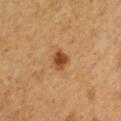workup: imaged on a skin check; not biopsied
subject: male, aged 48–52
tile lighting: cross-polarized
location: the right upper arm
acquisition: ~15 mm tile from a whole-body skin photo